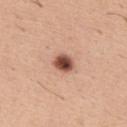Assessment:
This lesion was catalogued during total-body skin photography and was not selected for biopsy.
Acquisition and patient details:
The recorded lesion diameter is about 2.5 mm. The patient is a male approximately 40 years of age. The lesion is on the upper back. A roughly 15 mm field-of-view crop from a total-body skin photograph.